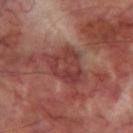Captured under cross-polarized illumination. The patient is a male aged around 70. A region of skin cropped from a whole-body photographic capture, roughly 15 mm wide. The lesion is on the left thigh. About 5.5 mm across.